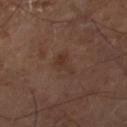The lesion was tiled from a total-body skin photograph and was not biopsied.
The lesion is located on the right thigh.
A 15 mm close-up tile from a total-body photography series done for melanoma screening.